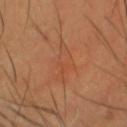No biopsy was performed on this lesion — it was imaged during a full skin examination and was not determined to be concerning. The tile uses cross-polarized illumination. Measured at roughly 5.5 mm in maximum diameter. The lesion-visualizer software estimated an area of roughly 7.5 mm², a shape eccentricity near 0.9, and a shape-asymmetry score of about 0.45 (0 = symmetric). The software also gave an average lesion color of about L≈47 a*≈25 b*≈34 (CIELAB), about 4 CIELAB-L* units darker than the surrounding skin, and a normalized border contrast of about 3.5. The analysis additionally found a border-irregularity index near 6.5/10 and a within-lesion color-variation index near 2.5/10. A male subject, aged 43–47. The lesion is located on the head or neck. A 15 mm close-up tile from a total-body photography series done for melanoma screening.